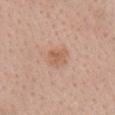No biopsy was performed on this lesion — it was imaged during a full skin examination and was not determined to be concerning.
A close-up tile cropped from a whole-body skin photograph, about 15 mm across.
From the head or neck.
Imaged with white-light lighting.
A female patient aged around 25.